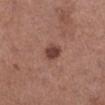Background:
A female patient approximately 60 years of age. On the left lower leg. The recorded lesion diameter is about 2.5 mm. This is a white-light tile. A 15 mm crop from a total-body photograph taken for skin-cancer surveillance. Automated tile analysis of the lesion measured an area of roughly 4.5 mm², an eccentricity of roughly 0.6, and a shape-asymmetry score of about 0.15 (0 = symmetric). And it measured a classifier nevus-likeness of about 90/100 and a lesion-detection confidence of about 100/100.